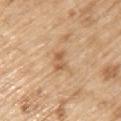notes = total-body-photography surveillance lesion; no biopsy | anatomic site = the left upper arm | TBP lesion metrics = an area of roughly 3.5 mm² and a symmetry-axis asymmetry near 0.25; a lesion color around L≈59 a*≈20 b*≈37 in CIELAB and a lesion–skin lightness drop of about 10; an automated nevus-likeness rating near 0 out of 100 and lesion-presence confidence of about 100/100 | acquisition = 15 mm crop, total-body photography | lesion diameter = ≈2.5 mm | lighting = white-light illumination | patient = male, about 70 years old.